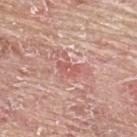Q: Was a biopsy performed?
A: imaged on a skin check; not biopsied
Q: How large is the lesion?
A: ≈3 mm
Q: Lesion location?
A: the back
Q: Who is the patient?
A: male, in their mid- to late 60s
Q: What kind of image is this?
A: total-body-photography crop, ~15 mm field of view